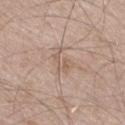follow-up = imaged on a skin check; not biopsied | imaging modality = ~15 mm tile from a whole-body skin photo | patient = male, roughly 65 years of age | automated metrics = two-axis asymmetry of about 0.55; an average lesion color of about L≈59 a*≈16 b*≈27 (CIELAB) and about 7 CIELAB-L* units darker than the surrounding skin; a border-irregularity rating of about 8/10 and a color-variation rating of about 1/10; a classifier nevus-likeness of about 0/100 and a lesion-detection confidence of about 95/100 | illumination = white-light | diameter = about 3.5 mm | location = the right thigh.